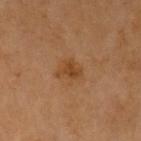Assessment: This lesion was catalogued during total-body skin photography and was not selected for biopsy. Clinical summary: A male subject, aged 68–72. Cropped from a total-body skin-imaging series; the visible field is about 15 mm. The lesion is located on the left upper arm. The total-body-photography lesion software estimated a footprint of about 4 mm², an eccentricity of roughly 0.7, and two-axis asymmetry of about 0.35. And it measured a color-variation rating of about 1.5/10 and radial color variation of about 0.5.